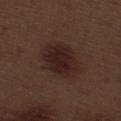Impression: The lesion was photographed on a routine skin check and not biopsied; there is no pathology result. Image and clinical context: The lesion is on the right thigh. Approximately 5.5 mm at its widest. A 15 mm close-up extracted from a 3D total-body photography capture. The patient is a male approximately 70 years of age. The tile uses white-light illumination.